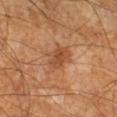Case summary:
* diameter: about 4 mm
* subject: male, roughly 60 years of age
* lighting: cross-polarized
* imaging modality: total-body-photography crop, ~15 mm field of view
* site: the leg